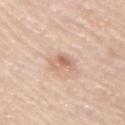workup: catalogued during a skin exam; not biopsied | subject: male, aged 53–57 | image: ~15 mm tile from a whole-body skin photo | automated metrics: a lesion area of about 5 mm² and a shape-asymmetry score of about 0.25 (0 = symmetric) | site: the right upper arm.